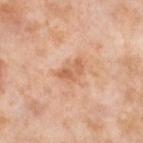workup — imaged on a skin check; not biopsied
TBP lesion metrics — a mean CIELAB color near L≈63 a*≈24 b*≈35, a lesion–skin lightness drop of about 9, and a normalized border contrast of about 6
lesion diameter — about 3.5 mm
body site — the left thigh
image — ~15 mm tile from a whole-body skin photo
lighting — cross-polarized
patient — female, in their mid- to late 50s